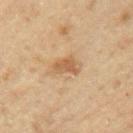Acquisition and patient details: The subject is a male aged 43 to 47. A lesion tile, about 15 mm wide, cut from a 3D total-body photograph. The lesion is located on the left upper arm.